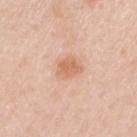This lesion was catalogued during total-body skin photography and was not selected for biopsy. The total-body-photography lesion software estimated a footprint of about 6 mm², an eccentricity of roughly 0.6, and a shape-asymmetry score of about 0.25 (0 = symmetric). It also reported an average lesion color of about L≈66 a*≈23 b*≈34 (CIELAB), roughly 9 lightness units darker than nearby skin, and a normalized border contrast of about 6.5. The software also gave a detector confidence of about 100 out of 100 that the crop contains a lesion. A 15 mm close-up extracted from a 3D total-body photography capture. Longest diameter approximately 3 mm. The subject is a male aged 58 to 62. From the left upper arm. Captured under white-light illumination.